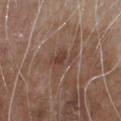Notes:
- body site · the front of the torso
- acquisition · ~15 mm crop, total-body skin-cancer survey
- patient · male, aged 78 to 82